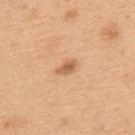Case summary:
– follow-up · total-body-photography surveillance lesion; no biopsy